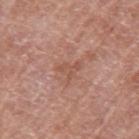Impression: This lesion was catalogued during total-body skin photography and was not selected for biopsy. Clinical summary: A female patient aged approximately 60. The lesion's longest dimension is about 3 mm. Captured under white-light illumination. Located on the left upper arm. A roughly 15 mm field-of-view crop from a total-body skin photograph. The lesion-visualizer software estimated a footprint of about 3 mm² and a symmetry-axis asymmetry near 0.7. The analysis additionally found a color-variation rating of about 0/10 and peripheral color asymmetry of about 0.